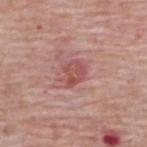The lesion is located on the back. The lesion's longest dimension is about 3 mm. Captured under white-light illumination. A male subject, roughly 65 years of age. A region of skin cropped from a whole-body photographic capture, roughly 15 mm wide.